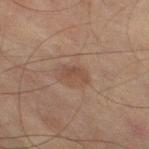- workup: catalogued during a skin exam; not biopsied
- automated lesion analysis: about 6 CIELAB-L* units darker than the surrounding skin and a normalized border contrast of about 5.5; border irregularity of about 4 on a 0–10 scale, internal color variation of about 1 on a 0–10 scale, and peripheral color asymmetry of about 0.5; a classifier nevus-likeness of about 15/100 and a lesion-detection confidence of about 100/100
- illumination: cross-polarized illumination
- subject: male, approximately 75 years of age
- location: the right thigh
- image: total-body-photography crop, ~15 mm field of view
- size: ~2.5 mm (longest diameter)